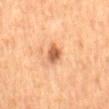Findings:
– site — the mid back
– subject — female, in their 60s
– tile lighting — cross-polarized illumination
– acquisition — ~15 mm tile from a whole-body skin photo
– image-analysis metrics — a border-irregularity rating of about 2.5/10, internal color variation of about 3.5 on a 0–10 scale, and peripheral color asymmetry of about 1; a nevus-likeness score of about 85/100 and a lesion-detection confidence of about 100/100